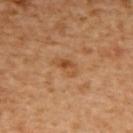This lesion was catalogued during total-body skin photography and was not selected for biopsy. The lesion-visualizer software estimated a lesion area of about 4 mm² and an outline eccentricity of about 0.85 (0 = round, 1 = elongated). It also reported an average lesion color of about L≈51 a*≈24 b*≈39 (CIELAB) and a normalized lesion–skin contrast near 6.5. And it measured a border-irregularity index near 3/10 and radial color variation of about 2. It also reported a nevus-likeness score of about 40/100 and a lesion-detection confidence of about 100/100. Longest diameter approximately 3 mm. Imaged with cross-polarized lighting. A female patient roughly 55 years of age. A lesion tile, about 15 mm wide, cut from a 3D total-body photograph. From the upper back.